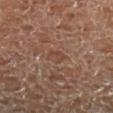Recorded during total-body skin imaging; not selected for excision or biopsy. From the left lower leg. The tile uses cross-polarized illumination. About 2.5 mm across. Cropped from a whole-body photographic skin survey; the tile spans about 15 mm. A male subject in their mid-50s.